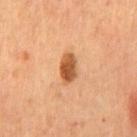Findings:
– workup · catalogued during a skin exam; not biopsied
– site · the front of the torso
– image source · 15 mm crop, total-body photography
– lighting · cross-polarized
– lesion diameter · ~3.5 mm (longest diameter)
– patient · male, aged approximately 55